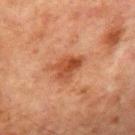Recorded during total-body skin imaging; not selected for excision or biopsy. A 15 mm close-up tile from a total-body photography series done for melanoma screening. Located on the chest. Measured at roughly 4 mm in maximum diameter. This is a cross-polarized tile. The patient is a male approximately 65 years of age.